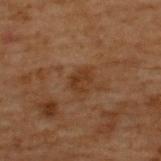biopsy_status: not biopsied; imaged during a skin examination
image:
  source: total-body photography crop
  field_of_view_mm: 15
lighting: cross-polarized
site: back
lesion_size:
  long_diameter_mm_approx: 2.5
patient:
  sex: male
  age_approx: 70
automated_metrics:
  area_mm2_approx: 4.0
  eccentricity: 0.7
  shape_asymmetry: 0.25
  cielab_L: 26
  cielab_a: 16
  cielab_b: 25
  vs_skin_contrast_norm: 6.5
  border_irregularity_0_10: 2.0
  color_variation_0_10: 2.0
  peripheral_color_asymmetry: 0.5
  nevus_likeness_0_100: 0
  lesion_detection_confidence_0_100: 100